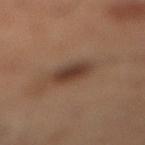workup = imaged on a skin check; not biopsied | imaging modality = ~15 mm crop, total-body skin-cancer survey | lighting = cross-polarized illumination | diameter = ≈3 mm | image-analysis metrics = a lesion area of about 6.5 mm² and an eccentricity of roughly 0.65; a lesion color around L≈37 a*≈18 b*≈25 in CIELAB and roughly 10 lightness units darker than nearby skin; a border-irregularity index near 1.5/10 and radial color variation of about 1; a classifier nevus-likeness of about 90/100 | patient = male, approximately 60 years of age | anatomic site = the right lower leg.